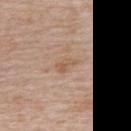The lesion was photographed on a routine skin check and not biopsied; there is no pathology result. The lesion's longest dimension is about 2.5 mm. A 15 mm close-up tile from a total-body photography series done for melanoma screening. Imaged with white-light lighting. The lesion-visualizer software estimated a lesion area of about 3.5 mm², a shape eccentricity near 0.8, and a shape-asymmetry score of about 0.35 (0 = symmetric). The analysis additionally found an average lesion color of about L≈57 a*≈17 b*≈32 (CIELAB), about 7 CIELAB-L* units darker than the surrounding skin, and a normalized border contrast of about 6. It also reported a border-irregularity rating of about 3/10 and a peripheral color-asymmetry measure near 1.5. The software also gave a nevus-likeness score of about 0/100 and a detector confidence of about 100 out of 100 that the crop contains a lesion. The patient is a female aged 48–52. On the upper back.